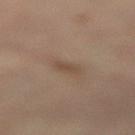follow-up = total-body-photography surveillance lesion; no biopsy | size = about 3 mm | subject = female, aged around 65 | imaging modality = 15 mm crop, total-body photography | location = the right lower leg | tile lighting = cross-polarized illumination.